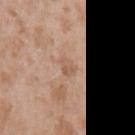follow-up = total-body-photography surveillance lesion; no biopsy
illumination = white-light illumination
patient = male, aged 48 to 52
automated lesion analysis = a footprint of about 4 mm², a shape eccentricity near 0.6, and two-axis asymmetry of about 0.4; border irregularity of about 3.5 on a 0–10 scale and a color-variation rating of about 3/10; a nevus-likeness score of about 0/100 and a lesion-detection confidence of about 100/100
imaging modality = ~15 mm crop, total-body skin-cancer survey
location = the left upper arm
size = ~2.5 mm (longest diameter)